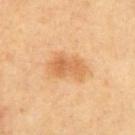* workup · total-body-photography surveillance lesion; no biopsy
* lighting · cross-polarized
* anatomic site · the chest
* image · 15 mm crop, total-body photography
* size · ~4.5 mm (longest diameter)
* subject · male, about 65 years old